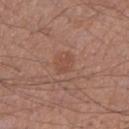This lesion was catalogued during total-body skin photography and was not selected for biopsy. A male patient in their mid-50s. On the right upper arm. Longest diameter approximately 2.5 mm. A close-up tile cropped from a whole-body skin photograph, about 15 mm across.